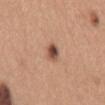Assessment:
The lesion was photographed on a routine skin check and not biopsied; there is no pathology result.
Image and clinical context:
Imaged with white-light lighting. A female subject roughly 30 years of age. The lesion's longest dimension is about 2.5 mm. Automated image analysis of the tile measured a lesion area of about 3.5 mm² and a shape-asymmetry score of about 0.2 (0 = symmetric). It also reported a lesion–skin lightness drop of about 15 and a normalized lesion–skin contrast near 10.5. And it measured a border-irregularity index near 1.5/10, a within-lesion color-variation index near 6.5/10, and radial color variation of about 2. The software also gave a classifier nevus-likeness of about 95/100 and a detector confidence of about 100 out of 100 that the crop contains a lesion. From the mid back. A 15 mm close-up extracted from a 3D total-body photography capture.